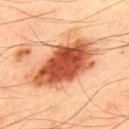Clinical impression: The lesion was tiled from a total-body skin photograph and was not biopsied. Background: Automated image analysis of the tile measured an area of roughly 30 mm², a shape eccentricity near 0.85, and a shape-asymmetry score of about 0.2 (0 = symmetric). The analysis additionally found a border-irregularity rating of about 3/10 and a peripheral color-asymmetry measure near 2.5. A 15 mm close-up tile from a total-body photography series done for melanoma screening. The subject is a male approximately 50 years of age. From the upper back. Measured at roughly 8.5 mm in maximum diameter.